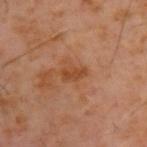Q: Was a biopsy performed?
A: imaged on a skin check; not biopsied
Q: What is the lesion's diameter?
A: ≈3 mm
Q: What is the imaging modality?
A: ~15 mm crop, total-body skin-cancer survey
Q: What are the patient's age and sex?
A: male, roughly 60 years of age
Q: How was the tile lit?
A: cross-polarized
Q: Where on the body is the lesion?
A: the upper back